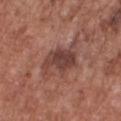Recorded during total-body skin imaging; not selected for excision or biopsy. The tile uses white-light illumination. The patient is a male approximately 75 years of age. A 15 mm crop from a total-body photograph taken for skin-cancer surveillance. Located on the front of the torso.